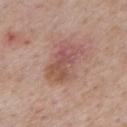• workup — imaged on a skin check; not biopsied
• patient — male, in their mid- to late 70s
• acquisition — ~15 mm crop, total-body skin-cancer survey
• anatomic site — the mid back
• illumination — white-light
• automated lesion analysis — an area of roughly 12 mm², an eccentricity of roughly 0.85, and a shape-asymmetry score of about 0.2 (0 = symmetric); an average lesion color of about L≈53 a*≈22 b*≈25 (CIELAB), a lesion–skin lightness drop of about 9, and a normalized border contrast of about 6.5; a detector confidence of about 100 out of 100 that the crop contains a lesion
• lesion diameter — ~5 mm (longest diameter)